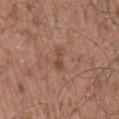Impression: The lesion was tiled from a total-body skin photograph and was not biopsied. Image and clinical context: A male patient about 55 years old. A lesion tile, about 15 mm wide, cut from a 3D total-body photograph. Automated tile analysis of the lesion measured an average lesion color of about L≈47 a*≈21 b*≈28 (CIELAB), about 7 CIELAB-L* units darker than the surrounding skin, and a normalized border contrast of about 6. The lesion is on the back. The recorded lesion diameter is about 3 mm.